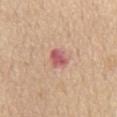The lesion's longest dimension is about 3 mm. The lesion is located on the chest. The tile uses white-light illumination. A male subject, aged 68–72. A 15 mm close-up extracted from a 3D total-body photography capture. The total-body-photography lesion software estimated a footprint of about 5 mm², a shape eccentricity near 0.75, and a shape-asymmetry score of about 0.2 (0 = symmetric). And it measured a lesion–skin lightness drop of about 12 and a normalized border contrast of about 9.